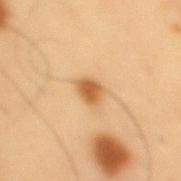| field | value |
|---|---|
| follow-up | total-body-photography surveillance lesion; no biopsy |
| tile lighting | cross-polarized illumination |
| image source | ~15 mm tile from a whole-body skin photo |
| TBP lesion metrics | a lesion color around L≈58 a*≈22 b*≈42 in CIELAB, a lesion–skin lightness drop of about 13, and a normalized lesion–skin contrast near 9 |
| subject | male, roughly 55 years of age |
| location | the back |